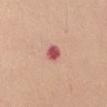Case summary:
- follow-up · total-body-photography surveillance lesion; no biopsy